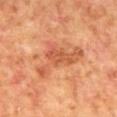Context:
A roughly 15 mm field-of-view crop from a total-body skin photograph. Located on the back. A male subject, in their 70s. Captured under cross-polarized illumination.